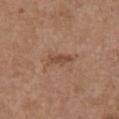workup: catalogued during a skin exam; not biopsied | patient: female, approximately 65 years of age | image source: ~15 mm crop, total-body skin-cancer survey | anatomic site: the chest | lesion diameter: ≈3 mm | automated lesion analysis: a lesion area of about 4 mm² and a shape-asymmetry score of about 0.25 (0 = symmetric); a lesion color around L≈48 a*≈20 b*≈30 in CIELAB, about 8 CIELAB-L* units darker than the surrounding skin, and a normalized border contrast of about 6.5; a nevus-likeness score of about 10/100 and a lesion-detection confidence of about 100/100.